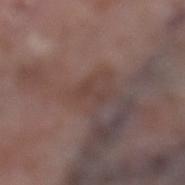From the left lower leg. This is a white-light tile. The subject is a female roughly 70 years of age. The recorded lesion diameter is about 2.5 mm. Cropped from a total-body skin-imaging series; the visible field is about 15 mm.